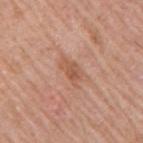The lesion was photographed on a routine skin check and not biopsied; there is no pathology result. Measured at roughly 3.5 mm in maximum diameter. The subject is a male aged 48 to 52. A 15 mm crop from a total-body photograph taken for skin-cancer surveillance. Captured under white-light illumination. The lesion is located on the right upper arm.